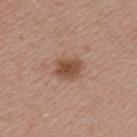Notes:
* workup · total-body-photography surveillance lesion; no biopsy
* acquisition · ~15 mm tile from a whole-body skin photo
* body site · the left upper arm
* size · about 3.5 mm
* tile lighting · white-light illumination
* subject · female, approximately 45 years of age